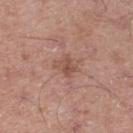Case summary:
- follow-up · catalogued during a skin exam; not biopsied
- patient · male, aged 53 to 57
- image-analysis metrics · a shape eccentricity near 0.65 and a symmetry-axis asymmetry near 0.2; a mean CIELAB color near L≈51 a*≈21 b*≈27, roughly 8 lightness units darker than nearby skin, and a lesion-to-skin contrast of about 6 (normalized; higher = more distinct); a border-irregularity rating of about 2.5/10 and a within-lesion color-variation index near 3.5/10
- lighting · white-light
- image · ~15 mm tile from a whole-body skin photo
- diameter · ≈2.5 mm
- location · the right thigh